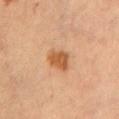The patient is a female roughly 60 years of age. The tile uses cross-polarized illumination. Located on the chest. About 3.5 mm across. A lesion tile, about 15 mm wide, cut from a 3D total-body photograph.